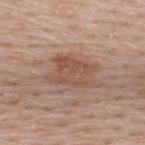Findings:
– follow-up: total-body-photography surveillance lesion; no biopsy
– illumination: white-light illumination
– image source: 15 mm crop, total-body photography
– image-analysis metrics: a footprint of about 14 mm²
– size: ≈5.5 mm
– body site: the upper back
– patient: male, aged around 60